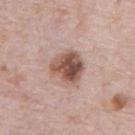Part of a total-body skin-imaging series; this lesion was reviewed on a skin check and was not flagged for biopsy. Located on the abdomen. The subject is a male aged approximately 70. A region of skin cropped from a whole-body photographic capture, roughly 15 mm wide.